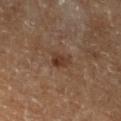Assessment: Captured during whole-body skin photography for melanoma surveillance; the lesion was not biopsied. Image and clinical context: The lesion's longest dimension is about 2.5 mm. A 15 mm crop from a total-body photograph taken for skin-cancer surveillance. On the left lower leg. A male patient, aged around 85 or older.